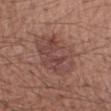image source: ~15 mm crop, total-body skin-cancer survey | body site: the right forearm | image-analysis metrics: a footprint of about 21 mm², a shape eccentricity near 0.75, and two-axis asymmetry of about 0.15; a border-irregularity rating of about 2.5/10 and internal color variation of about 5 on a 0–10 scale | subject: male, approximately 55 years of age.